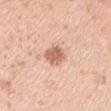Impression: Imaged during a routine full-body skin examination; the lesion was not biopsied and no histopathology is available. Acquisition and patient details: The subject is a male aged 28–32. On the left upper arm. A 15 mm close-up tile from a total-body photography series done for melanoma screening. An algorithmic analysis of the crop reported a within-lesion color-variation index near 3/10 and a peripheral color-asymmetry measure near 1. The analysis additionally found a classifier nevus-likeness of about 90/100 and a lesion-detection confidence of about 100/100. Longest diameter approximately 3 mm. The tile uses white-light illumination.